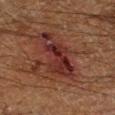workup = imaged on a skin check; not biopsied | subject = male, aged approximately 60 | anatomic site = the right lower leg | image = 15 mm crop, total-body photography.